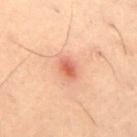workup: imaged on a skin check; not biopsied
anatomic site: the front of the torso
diameter: ≈2.5 mm
automated lesion analysis: a lesion area of about 4 mm², an eccentricity of roughly 0.7, and a shape-asymmetry score of about 0.15 (0 = symmetric); a mean CIELAB color near L≈52 a*≈26 b*≈30, a lesion–skin lightness drop of about 10, and a lesion-to-skin contrast of about 7.5 (normalized; higher = more distinct); a border-irregularity rating of about 1.5/10; an automated nevus-likeness rating near 70 out of 100
imaging modality: ~15 mm crop, total-body skin-cancer survey
patient: female, in their mid- to late 50s